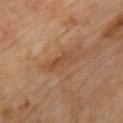Q: How was the tile lit?
A: cross-polarized
Q: What is the imaging modality?
A: ~15 mm tile from a whole-body skin photo
Q: Who is the patient?
A: male, roughly 70 years of age
Q: Automated lesion metrics?
A: border irregularity of about 4.5 on a 0–10 scale, a within-lesion color-variation index near 1.5/10, and radial color variation of about 0
Q: What is the lesion's diameter?
A: ~3.5 mm (longest diameter)
Q: Lesion location?
A: the chest